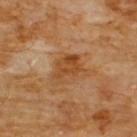Q: Was a biopsy performed?
A: imaged on a skin check; not biopsied
Q: Automated lesion metrics?
A: a footprint of about 8.5 mm², an outline eccentricity of about 0.65 (0 = round, 1 = elongated), and a symmetry-axis asymmetry near 0.35; an average lesion color of about L≈45 a*≈22 b*≈38 (CIELAB), about 8 CIELAB-L* units darker than the surrounding skin, and a normalized border contrast of about 7.5; a peripheral color-asymmetry measure near 1.5
Q: Illumination type?
A: cross-polarized
Q: What are the patient's age and sex?
A: male, roughly 60 years of age
Q: Where on the body is the lesion?
A: the chest
Q: What is the imaging modality?
A: total-body-photography crop, ~15 mm field of view